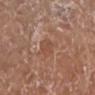Imaged during a routine full-body skin examination; the lesion was not biopsied and no histopathology is available. About 2.5 mm across. A female patient, about 75 years old. A 15 mm close-up tile from a total-body photography series done for melanoma screening. The lesion is located on the leg.